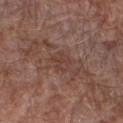The lesion was tiled from a total-body skin photograph and was not biopsied. A male patient in their 70s. A region of skin cropped from a whole-body photographic capture, roughly 15 mm wide. Located on the right forearm. Captured under white-light illumination.